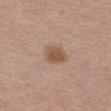The lesion was photographed on a routine skin check and not biopsied; there is no pathology result. Cropped from a whole-body photographic skin survey; the tile spans about 15 mm. The lesion is located on the chest. A female subject about 30 years old. The lesion's longest dimension is about 3 mm. An algorithmic analysis of the crop reported a lesion area of about 6.5 mm² and an eccentricity of roughly 0.65. And it measured a nevus-likeness score of about 90/100 and a lesion-detection confidence of about 100/100.